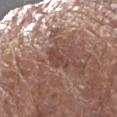The lesion is located on the right forearm.
Automated tile analysis of the lesion measured a mean CIELAB color near L≈44 a*≈19 b*≈24, about 7 CIELAB-L* units darker than the surrounding skin, and a normalized lesion–skin contrast near 6. It also reported a color-variation rating of about 1.5/10 and a peripheral color-asymmetry measure near 0.5.
The recorded lesion diameter is about 3.5 mm.
This image is a 15 mm lesion crop taken from a total-body photograph.
This is a white-light tile.
A female patient, about 80 years old.